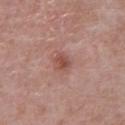Assessment:
The lesion was tiled from a total-body skin photograph and was not biopsied.
Clinical summary:
A male subject aged around 70. A 15 mm close-up tile from a total-body photography series done for melanoma screening. Imaged with white-light lighting. Longest diameter approximately 2.5 mm. The lesion is located on the chest. Automated image analysis of the tile measured a lesion area of about 4 mm², a shape eccentricity near 0.75, and a shape-asymmetry score of about 0.25 (0 = symmetric). The analysis additionally found roughly 9 lightness units darker than nearby skin and a normalized lesion–skin contrast near 7. The software also gave a classifier nevus-likeness of about 0/100 and a lesion-detection confidence of about 100/100.